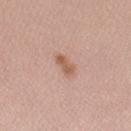Background:
The lesion is located on the left forearm. Longest diameter approximately 3 mm. Automated image analysis of the tile measured a classifier nevus-likeness of about 60/100 and lesion-presence confidence of about 100/100. A 15 mm close-up tile from a total-body photography series done for melanoma screening. A female subject, about 40 years old. Captured under white-light illumination.